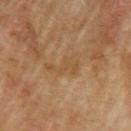biopsy status: imaged on a skin check; not biopsied | anatomic site: the left upper arm | automated metrics: an area of roughly 6.5 mm², a shape eccentricity near 0.85, and two-axis asymmetry of about 0.55 | lighting: cross-polarized illumination | imaging modality: total-body-photography crop, ~15 mm field of view | lesion diameter: about 4 mm | subject: male, roughly 75 years of age.